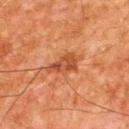notes: imaged on a skin check; not biopsied | lighting: cross-polarized illumination | subject: male, about 65 years old | body site: the upper back | image-analysis metrics: an area of roughly 6 mm², an eccentricity of roughly 0.8, and a shape-asymmetry score of about 0.4 (0 = symmetric); an average lesion color of about L≈40 a*≈24 b*≈33 (CIELAB); an automated nevus-likeness rating near 10 out of 100 | image source: ~15 mm tile from a whole-body skin photo | lesion size: ~3.5 mm (longest diameter).